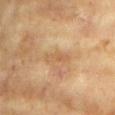Q: Was a biopsy performed?
A: no biopsy performed (imaged during a skin exam)
Q: What kind of image is this?
A: ~15 mm crop, total-body skin-cancer survey
Q: Lesion location?
A: the left upper arm
Q: What is the lesion's diameter?
A: about 3.5 mm
Q: Illumination type?
A: cross-polarized
Q: Who is the patient?
A: female, aged 73 to 77
Q: Automated lesion metrics?
A: an average lesion color of about L≈60 a*≈19 b*≈39 (CIELAB) and a normalized border contrast of about 5; a border-irregularity index near 3.5/10, a color-variation rating of about 0/10, and a peripheral color-asymmetry measure near 0